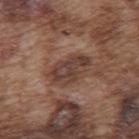A 15 mm close-up extracted from a 3D total-body photography capture. From the upper back. A male patient, roughly 75 years of age.